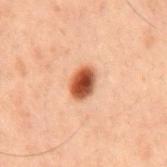Assessment:
The lesion was tiled from a total-body skin photograph and was not biopsied.
Clinical summary:
A male subject aged approximately 60. A 15 mm close-up extracted from a 3D total-body photography capture. The tile uses cross-polarized illumination. From the mid back.